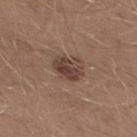Case summary:
- notes — no biopsy performed (imaged during a skin exam)
- lighting — white-light illumination
- image — ~15 mm crop, total-body skin-cancer survey
- lesion size — ≈4 mm
- subject — male, aged around 30
- location — the left lower leg